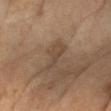Located on the right forearm. A 15 mm close-up extracted from a 3D total-body photography capture. The lesion's longest dimension is about 4.5 mm. A female subject, about 70 years old.